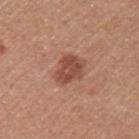This lesion was catalogued during total-body skin photography and was not selected for biopsy. On the left upper arm. The patient is a female aged around 50. Cropped from a total-body skin-imaging series; the visible field is about 15 mm. The total-body-photography lesion software estimated a lesion color around L≈49 a*≈24 b*≈29 in CIELAB and a lesion-to-skin contrast of about 8 (normalized; higher = more distinct). It also reported a border-irregularity rating of about 2.5/10, a color-variation rating of about 2.5/10, and a peripheral color-asymmetry measure near 1. It also reported a classifier nevus-likeness of about 75/100. The lesion's longest dimension is about 3.5 mm.